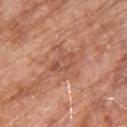Notes:
– biopsy status · imaged on a skin check; not biopsied
– subject · male, in their 80s
– lighting · white-light
– acquisition · ~15 mm crop, total-body skin-cancer survey
– anatomic site · the upper back
– lesion size · ~3.5 mm (longest diameter)